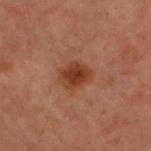Impression: Captured during whole-body skin photography for melanoma surveillance; the lesion was not biopsied. Clinical summary: On the head or neck. This image is a 15 mm lesion crop taken from a total-body photograph. Imaged with cross-polarized lighting. Automated image analysis of the tile measured border irregularity of about 2 on a 0–10 scale and radial color variation of about 1. A male patient, aged 58–62. The recorded lesion diameter is about 3 mm.